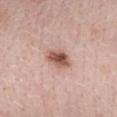No biopsy was performed on this lesion — it was imaged during a full skin examination and was not determined to be concerning.